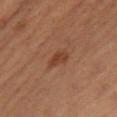workup: total-body-photography surveillance lesion; no biopsy | image: ~15 mm crop, total-body skin-cancer survey | patient: female, roughly 50 years of age | tile lighting: cross-polarized illumination | lesion size: ≈2.5 mm | anatomic site: the right thigh | TBP lesion metrics: an automated nevus-likeness rating near 75 out of 100 and a detector confidence of about 100 out of 100 that the crop contains a lesion.